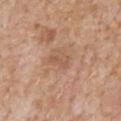This lesion was catalogued during total-body skin photography and was not selected for biopsy.
A close-up tile cropped from a whole-body skin photograph, about 15 mm across.
From the chest.
The tile uses white-light illumination.
A male subject, roughly 60 years of age.
Automated image analysis of the tile measured an area of roughly 3 mm², an outline eccentricity of about 0.85 (0 = round, 1 = elongated), and a symmetry-axis asymmetry near 0.45. And it measured about 7 CIELAB-L* units darker than the surrounding skin and a lesion-to-skin contrast of about 5 (normalized; higher = more distinct). The analysis additionally found border irregularity of about 4.5 on a 0–10 scale and radial color variation of about 0. And it measured an automated nevus-likeness rating near 0 out of 100.
Longest diameter approximately 2.5 mm.